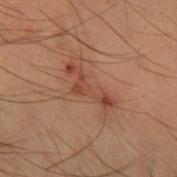Part of a total-body skin-imaging series; this lesion was reviewed on a skin check and was not flagged for biopsy. An algorithmic analysis of the crop reported an area of roughly 6.5 mm² and two-axis asymmetry of about 0.65. And it measured a nevus-likeness score of about 0/100 and a detector confidence of about 100 out of 100 that the crop contains a lesion. The lesion is on the arm. This is a cross-polarized tile. A 15 mm crop from a total-body photograph taken for skin-cancer surveillance. A male subject, approximately 55 years of age. About 5.5 mm across.